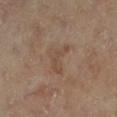Impression:
Captured during whole-body skin photography for melanoma surveillance; the lesion was not biopsied.
Background:
The lesion-visualizer software estimated a footprint of about 6.5 mm², an eccentricity of roughly 0.75, and a shape-asymmetry score of about 0.65 (0 = symmetric). The analysis additionally found a border-irregularity rating of about 6.5/10, a within-lesion color-variation index near 2/10, and peripheral color asymmetry of about 0.5. A lesion tile, about 15 mm wide, cut from a 3D total-body photograph. Approximately 4 mm at its widest. A male patient, roughly 65 years of age. From the left lower leg. Imaged with cross-polarized lighting.